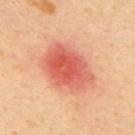biopsy_status: not biopsied; imaged during a skin examination
lighting: cross-polarized
image:
  source: total-body photography crop
  field_of_view_mm: 15
lesion_size:
  long_diameter_mm_approx: 6.0
site: upper back
patient:
  sex: male
  age_approx: 40